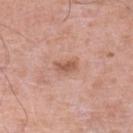– workup · total-body-photography surveillance lesion; no biopsy
– location · the leg
– patient · male, in their 80s
– diameter · ~2.5 mm (longest diameter)
– image · 15 mm crop, total-body photography
– illumination · white-light